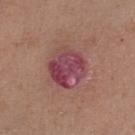This lesion was catalogued during total-body skin photography and was not selected for biopsy.
Located on the left lower leg.
Longest diameter approximately 4.5 mm.
Cropped from a total-body skin-imaging series; the visible field is about 15 mm.
An algorithmic analysis of the crop reported a lesion area of about 16 mm², an eccentricity of roughly 0.3, and two-axis asymmetry of about 0.15. The software also gave an automated nevus-likeness rating near 0 out of 100 and a lesion-detection confidence of about 100/100.
Imaged with white-light lighting.
The patient is a female aged approximately 40.